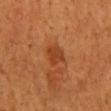The lesion was tiled from a total-body skin photograph and was not biopsied.
The lesion is on the mid back.
The subject is a male in their mid- to late 40s.
Imaged with cross-polarized lighting.
Cropped from a total-body skin-imaging series; the visible field is about 15 mm.
The lesion's longest dimension is about 3 mm.